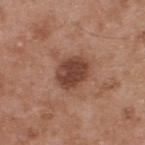| field | value |
|---|---|
| notes | total-body-photography surveillance lesion; no biopsy |
| body site | the upper back |
| lighting | white-light |
| diameter | ≈4 mm |
| patient | male, in their mid-50s |
| image | total-body-photography crop, ~15 mm field of view |